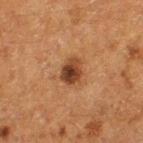Q: Was a biopsy performed?
A: no biopsy performed (imaged during a skin exam)
Q: What lighting was used for the tile?
A: cross-polarized
Q: What is the imaging modality?
A: 15 mm crop, total-body photography
Q: Patient demographics?
A: female, aged 48–52
Q: How large is the lesion?
A: ~3 mm (longest diameter)
Q: Lesion location?
A: the left thigh